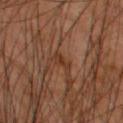biopsy status: no biopsy performed (imaged during a skin exam)
imaging modality: 15 mm crop, total-body photography
anatomic site: the upper back
patient: male, aged approximately 60